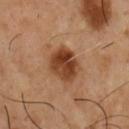follow-up=imaged on a skin check; not biopsied | tile lighting=cross-polarized illumination | image source=total-body-photography crop, ~15 mm field of view | location=the chest | lesion size=about 4.5 mm | subject=male, in their mid- to late 50s.